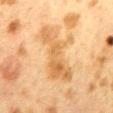follow-up = imaged on a skin check; not biopsied
body site = the mid back
tile lighting = cross-polarized
subject = female, aged approximately 40
lesion diameter = ≈7.5 mm
image source = total-body-photography crop, ~15 mm field of view